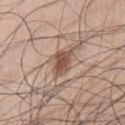biopsy_status: not biopsied; imaged during a skin examination
site: chest
image:
  source: total-body photography crop
  field_of_view_mm: 15
patient:
  sex: male
  age_approx: 70
lighting: white-light